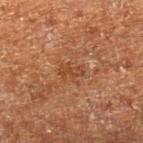The lesion was photographed on a routine skin check and not biopsied; there is no pathology result. From the right lower leg. The subject is a male aged 58 to 62. Longest diameter approximately 2.5 mm. Cropped from a total-body skin-imaging series; the visible field is about 15 mm. Imaged with cross-polarized lighting. The lesion-visualizer software estimated a border-irregularity index near 4/10 and internal color variation of about 0 on a 0–10 scale. The software also gave a nevus-likeness score of about 0/100 and a detector confidence of about 100 out of 100 that the crop contains a lesion.